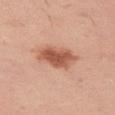This lesion was catalogued during total-body skin photography and was not selected for biopsy.